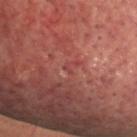Imaged during a routine full-body skin examination; the lesion was not biopsied and no histopathology is available. Captured under cross-polarized illumination. A lesion tile, about 15 mm wide, cut from a 3D total-body photograph. Longest diameter approximately 1.5 mm. Located on the head or neck. The lesion-visualizer software estimated an eccentricity of roughly 0.8 and a shape-asymmetry score of about 0.5 (0 = symmetric). The software also gave border irregularity of about 4.5 on a 0–10 scale, internal color variation of about 0 on a 0–10 scale, and radial color variation of about 0. The analysis additionally found a nevus-likeness score of about 0/100. A male patient aged around 55.